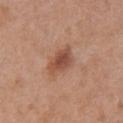{"biopsy_status": "not biopsied; imaged during a skin examination", "patient": {"sex": "female", "age_approx": 40}, "site": "back", "automated_metrics": {"area_mm2_approx": 7.5, "eccentricity": 0.8, "shape_asymmetry": 0.3, "cielab_L": 50, "cielab_a": 23, "cielab_b": 29, "vs_skin_contrast_norm": 8.0, "border_irregularity_0_10": 3.0, "color_variation_0_10": 4.0, "peripheral_color_asymmetry": 1.0, "nevus_likeness_0_100": 85}, "lesion_size": {"long_diameter_mm_approx": 4.0}, "image": {"source": "total-body photography crop", "field_of_view_mm": 15}}